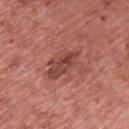follow-up: catalogued during a skin exam; not biopsied
automated lesion analysis: a lesion color around L≈46 a*≈27 b*≈26 in CIELAB, a lesion–skin lightness drop of about 9, and a normalized border contrast of about 7; a classifier nevus-likeness of about 10/100 and a detector confidence of about 100 out of 100 that the crop contains a lesion
location: the chest
size: about 5 mm
subject: male, in their mid-70s
image source: total-body-photography crop, ~15 mm field of view
lighting: white-light